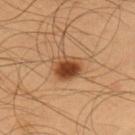{"biopsy_status": "not biopsied; imaged during a skin examination", "site": "left upper arm", "image": {"source": "total-body photography crop", "field_of_view_mm": 15}, "patient": {"sex": "male", "age_approx": 55}}